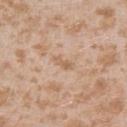Q: Is there a histopathology result?
A: imaged on a skin check; not biopsied